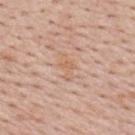Q: How large is the lesion?
A: ~3 mm (longest diameter)
Q: Automated lesion metrics?
A: an average lesion color of about L≈63 a*≈20 b*≈32 (CIELAB); a border-irregularity index near 4.5/10, a within-lesion color-variation index near 1/10, and peripheral color asymmetry of about 0.5
Q: Where on the body is the lesion?
A: the upper back
Q: What is the imaging modality?
A: total-body-photography crop, ~15 mm field of view
Q: What are the patient's age and sex?
A: male, roughly 40 years of age
Q: What lighting was used for the tile?
A: white-light